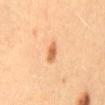The lesion was tiled from a total-body skin photograph and was not biopsied. Longest diameter approximately 3 mm. A region of skin cropped from a whole-body photographic capture, roughly 15 mm wide. The patient is a male about 40 years old. The lesion is on the lower back. Imaged with cross-polarized lighting.